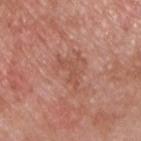{
  "biopsy_status": "not biopsied; imaged during a skin examination",
  "lighting": "white-light",
  "patient": {
    "sex": "male",
    "age_approx": 50
  },
  "automated_metrics": {
    "vs_skin_darker_L": 6.0,
    "vs_skin_contrast_norm": 5.0,
    "peripheral_color_asymmetry": 0.0
  },
  "image": {
    "source": "total-body photography crop",
    "field_of_view_mm": 15
  },
  "site": "front of the torso"
}